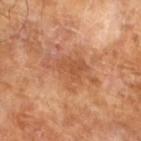{
  "biopsy_status": "not biopsied; imaged during a skin examination",
  "automated_metrics": {
    "area_mm2_approx": 12.0,
    "eccentricity": 0.8,
    "shape_asymmetry": 0.45
  },
  "patient": {
    "sex": "male",
    "age_approx": 65
  },
  "image": {
    "source": "total-body photography crop",
    "field_of_view_mm": 15
  },
  "lighting": "cross-polarized",
  "lesion_size": {
    "long_diameter_mm_approx": 6.0
  }
}